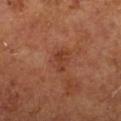Impression:
The lesion was tiled from a total-body skin photograph and was not biopsied.
Clinical summary:
The lesion is located on the right lower leg. An algorithmic analysis of the crop reported an outline eccentricity of about 0.9 (0 = round, 1 = elongated). And it measured a lesion color around L≈39 a*≈25 b*≈32 in CIELAB, a lesion–skin lightness drop of about 7, and a lesion-to-skin contrast of about 6 (normalized; higher = more distinct). And it measured a border-irregularity rating of about 5.5/10 and a peripheral color-asymmetry measure near 0. And it measured a nevus-likeness score of about 5/100. Cropped from a whole-body photographic skin survey; the tile spans about 15 mm. A male subject aged 63 to 67.